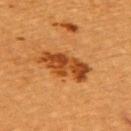Imaged during a routine full-body skin examination; the lesion was not biopsied and no histopathology is available. The total-body-photography lesion software estimated a lesion–skin lightness drop of about 12 and a normalized border contrast of about 9.5. It also reported a border-irregularity index near 3.5/10 and a color-variation rating of about 5.5/10. From the upper back. The tile uses cross-polarized illumination. A female subject, aged approximately 55. The recorded lesion diameter is about 6.5 mm. A 15 mm close-up extracted from a 3D total-body photography capture.